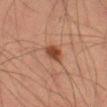This lesion was catalogued during total-body skin photography and was not selected for biopsy.
Automated image analysis of the tile measured a lesion area of about 4.5 mm², an eccentricity of roughly 0.65, and a shape-asymmetry score of about 0.3 (0 = symmetric). The software also gave a lesion color around L≈36 a*≈20 b*≈26 in CIELAB and roughly 10 lightness units darker than nearby skin.
The lesion is on the right thigh.
The tile uses cross-polarized illumination.
A male patient, in their mid- to late 60s.
Longest diameter approximately 3 mm.
Cropped from a total-body skin-imaging series; the visible field is about 15 mm.